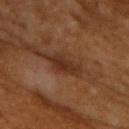– acquisition: 15 mm crop, total-body photography
– patient: male, in their mid-60s